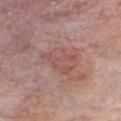The tile uses white-light illumination. A close-up tile cropped from a whole-body skin photograph, about 15 mm across. Measured at roughly 4.5 mm in maximum diameter. The subject is a male aged 58 to 62. The lesion is on the front of the torso.